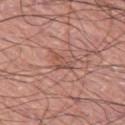Q: Is there a histopathology result?
A: catalogued during a skin exam; not biopsied
Q: How large is the lesion?
A: about 3 mm
Q: What is the anatomic site?
A: the left thigh
Q: What did automated image analysis measure?
A: an average lesion color of about L≈51 a*≈23 b*≈27 (CIELAB), roughly 8 lightness units darker than nearby skin, and a lesion-to-skin contrast of about 5.5 (normalized; higher = more distinct); a border-irregularity index near 6/10, a color-variation rating of about 0/10, and radial color variation of about 0; a nevus-likeness score of about 0/100 and lesion-presence confidence of about 55/100
Q: Illumination type?
A: white-light
Q: Patient demographics?
A: male, roughly 75 years of age
Q: What is the imaging modality?
A: ~15 mm crop, total-body skin-cancer survey